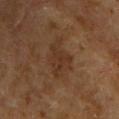Part of a total-body skin-imaging series; this lesion was reviewed on a skin check and was not flagged for biopsy. About 4.5 mm across. Cropped from a whole-body photographic skin survey; the tile spans about 15 mm. The tile uses cross-polarized illumination. The total-body-photography lesion software estimated a footprint of about 12 mm², an outline eccentricity of about 0.7 (0 = round, 1 = elongated), and a shape-asymmetry score of about 0.3 (0 = symmetric). It also reported a lesion color around L≈29 a*≈16 b*≈26 in CIELAB, roughly 5 lightness units darker than nearby skin, and a normalized lesion–skin contrast near 5.5. The lesion is on the upper back. A male subject aged around 65.